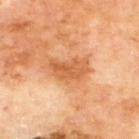– notes: imaged on a skin check; not biopsied
– body site: the back
– image source: 15 mm crop, total-body photography
– size: ~4.5 mm (longest diameter)
– illumination: cross-polarized illumination
– patient: male, in their 70s